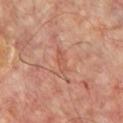  site: front of the torso
  automated_metrics:
    area_mm2_approx: 3.0
    eccentricity: 0.95
    peripheral_color_asymmetry: 0.0
  image:
    source: total-body photography crop
    field_of_view_mm: 15
  patient:
    sex: male
    age_approx: 55
  lighting: cross-polarized